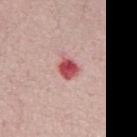Impression:
Imaged during a routine full-body skin examination; the lesion was not biopsied and no histopathology is available.
Context:
The lesion is located on the chest. Imaged with white-light lighting. This image is a 15 mm lesion crop taken from a total-body photograph. A male subject, roughly 65 years of age. The recorded lesion diameter is about 2.5 mm.